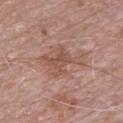Clinical impression: Captured during whole-body skin photography for melanoma surveillance; the lesion was not biopsied. Image and clinical context: A male patient approximately 70 years of age. The lesion's longest dimension is about 5 mm. Imaged with white-light lighting. A 15 mm close-up extracted from a 3D total-body photography capture. From the chest.